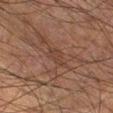Assessment: Captured during whole-body skin photography for melanoma surveillance; the lesion was not biopsied. Background: About 3 mm across. From the right lower leg. Imaged with cross-polarized lighting. A region of skin cropped from a whole-body photographic capture, roughly 15 mm wide. The patient is a male aged approximately 50.